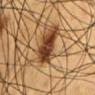Acquisition and patient details: The recorded lesion diameter is about 4 mm. The lesion is located on the chest. This is a cross-polarized tile. A lesion tile, about 15 mm wide, cut from a 3D total-body photograph. The subject is a male aged around 60. An algorithmic analysis of the crop reported a lesion area of about 8 mm², an outline eccentricity of about 0.8 (0 = round, 1 = elongated), and a shape-asymmetry score of about 0.3 (0 = symmetric). And it measured a mean CIELAB color near L≈38 a*≈23 b*≈34 and about 19 CIELAB-L* units darker than the surrounding skin. It also reported peripheral color asymmetry of about 1.5.